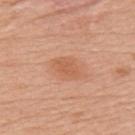• biopsy status · catalogued during a skin exam; not biopsied
• subject · female, roughly 50 years of age
• image source · ~15 mm tile from a whole-body skin photo
• TBP lesion metrics · an area of roughly 5 mm² and a symmetry-axis asymmetry near 0.2; an average lesion color of about L≈59 a*≈26 b*≈35 (CIELAB) and about 8 CIELAB-L* units darker than the surrounding skin; an automated nevus-likeness rating near 75 out of 100
• lighting · white-light
• size · ≈3 mm
• site · the upper back